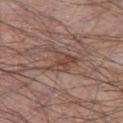Part of a total-body skin-imaging series; this lesion was reviewed on a skin check and was not flagged for biopsy. A male patient, in their mid-50s. The lesion-visualizer software estimated an area of roughly 7.5 mm², an eccentricity of roughly 0.55, and a shape-asymmetry score of about 0.5 (0 = symmetric). The software also gave a nevus-likeness score of about 5/100. A 15 mm crop from a total-body photograph taken for skin-cancer surveillance. About 4 mm across. The lesion is located on the leg. This is a white-light tile.